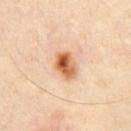Assessment:
No biopsy was performed on this lesion — it was imaged during a full skin examination and was not determined to be concerning.
Clinical summary:
On the chest. A 15 mm close-up tile from a total-body photography series done for melanoma screening. Measured at roughly 3.5 mm in maximum diameter. The subject is a male aged 33–37.